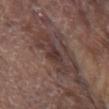– follow-up — total-body-photography surveillance lesion; no biopsy
– anatomic site — the chest
– imaging modality — 15 mm crop, total-body photography
– illumination — white-light
– patient — male, approximately 80 years of age
– size — ~2.5 mm (longest diameter)